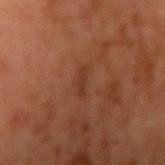follow-up = no biopsy performed (imaged during a skin exam)
location = the right upper arm
tile lighting = cross-polarized illumination
patient = male, in their 60s
lesion diameter = ≈3 mm
acquisition = ~15 mm tile from a whole-body skin photo